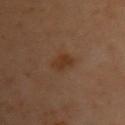Impression:
Part of a total-body skin-imaging series; this lesion was reviewed on a skin check and was not flagged for biopsy.
Acquisition and patient details:
The lesion is located on the chest. This image is a 15 mm lesion crop taken from a total-body photograph. Imaged with cross-polarized lighting. A female patient, in their 60s. Measured at roughly 3 mm in maximum diameter.